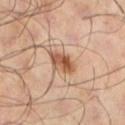Notes:
• biopsy status · no biopsy performed (imaged during a skin exam)
• subject · male, roughly 65 years of age
• acquisition · ~15 mm tile from a whole-body skin photo
• tile lighting · cross-polarized illumination
• automated metrics · a lesion area of about 6.5 mm², an outline eccentricity of about 0.8 (0 = round, 1 = elongated), and two-axis asymmetry of about 0.25
• anatomic site · the left lower leg